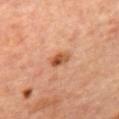The lesion was photographed on a routine skin check and not biopsied; there is no pathology result. The recorded lesion diameter is about 2.5 mm. The total-body-photography lesion software estimated a lesion color around L≈52 a*≈26 b*≈37 in CIELAB and a lesion-to-skin contrast of about 9 (normalized; higher = more distinct). And it measured a border-irregularity rating of about 2/10, internal color variation of about 8 on a 0–10 scale, and radial color variation of about 3. A female subject, approximately 45 years of age. This is a cross-polarized tile. A lesion tile, about 15 mm wide, cut from a 3D total-body photograph. The lesion is on the upper back.